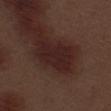Acquisition and patient details: A 15 mm close-up tile from a total-body photography series done for melanoma screening. A male subject, roughly 70 years of age. Imaged with white-light lighting. Measured at roughly 5.5 mm in maximum diameter. The lesion is located on the left thigh.